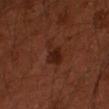<lesion>
<biopsy_status>not biopsied; imaged during a skin examination</biopsy_status>
<site>left upper arm</site>
<image>
  <source>total-body photography crop</source>
  <field_of_view_mm>15</field_of_view_mm>
</image>
<patient>
  <sex>male</sex>
  <age_approx>50</age_approx>
</patient>
</lesion>